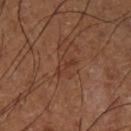The lesion was photographed on a routine skin check and not biopsied; there is no pathology result. The lesion is located on the leg. A 15 mm crop from a total-body photograph taken for skin-cancer surveillance. A male patient aged around 65. This is a cross-polarized tile.